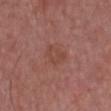Q: Was a biopsy performed?
A: no biopsy performed (imaged during a skin exam)
Q: What kind of image is this?
A: ~15 mm crop, total-body skin-cancer survey
Q: Lesion location?
A: the chest
Q: What are the patient's age and sex?
A: male, aged approximately 65
Q: Lesion size?
A: ≈3 mm